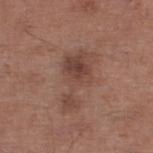  biopsy_status: not biopsied; imaged during a skin examination
  site: left lower leg
  patient:
    sex: male
    age_approx: 65
  automated_metrics:
    area_mm2_approx: 16.0
    eccentricity: 0.9
    shape_asymmetry: 0.5
    nevus_likeness_0_100: 25
    lesion_detection_confidence_0_100: 100
  lighting: white-light
  image:
    source: total-body photography crop
    field_of_view_mm: 15
  lesion_size:
    long_diameter_mm_approx: 7.0